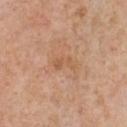lighting = white-light | acquisition = ~15 mm crop, total-body skin-cancer survey | body site = the chest | automated lesion analysis = border irregularity of about 5 on a 0–10 scale and a within-lesion color-variation index near 1/10; a nevus-likeness score of about 0/100 and a detector confidence of about 100 out of 100 that the crop contains a lesion | patient = male, aged approximately 60 | lesion diameter = ≈2.5 mm.